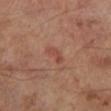Clinical impression:
Recorded during total-body skin imaging; not selected for excision or biopsy.
Clinical summary:
A 15 mm crop from a total-body photograph taken for skin-cancer surveillance. The lesion is located on the left lower leg. A male subject roughly 70 years of age. The lesion-visualizer software estimated a lesion color around L≈45 a*≈25 b*≈27 in CIELAB, a lesion–skin lightness drop of about 7, and a normalized border contrast of about 5.5. The analysis additionally found border irregularity of about 4 on a 0–10 scale and peripheral color asymmetry of about 0. And it measured a nevus-likeness score of about 15/100 and lesion-presence confidence of about 100/100. The tile uses cross-polarized illumination. The lesion's longest dimension is about 2.5 mm.